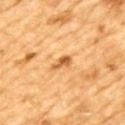<tbp_lesion>
<biopsy_status>not biopsied; imaged during a skin examination</biopsy_status>
<image>
  <source>total-body photography crop</source>
  <field_of_view_mm>15</field_of_view_mm>
</image>
<lighting>cross-polarized</lighting>
<site>mid back</site>
<automated_metrics>
  <eccentricity>0.9</eccentricity>
  <shape_asymmetry>0.35</shape_asymmetry>
  <border_irregularity_0_10>3.5</border_irregularity_0_10>
  <color_variation_0_10>1.5</color_variation_0_10>
</automated_metrics>
<patient>
  <sex>male</sex>
  <age_approx>85+</age_approx>
</patient>
<lesion_size>
  <long_diameter_mm_approx>3.0</long_diameter_mm_approx>
</lesion_size>
</tbp_lesion>